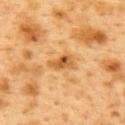Captured during whole-body skin photography for melanoma surveillance; the lesion was not biopsied. Longest diameter approximately 3 mm. The lesion-visualizer software estimated an area of roughly 4.5 mm², an eccentricity of roughly 0.8, and a symmetry-axis asymmetry near 0.35. The analysis additionally found roughly 10 lightness units darker than nearby skin and a normalized border contrast of about 8. Imaged with cross-polarized lighting. The patient is a female roughly 40 years of age. A roughly 15 mm field-of-view crop from a total-body skin photograph. Located on the upper back.